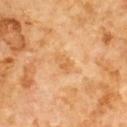This lesion was catalogued during total-body skin photography and was not selected for biopsy. This image is a 15 mm lesion crop taken from a total-body photograph. On the chest. The subject is a male approximately 60 years of age.